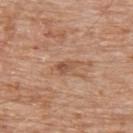The lesion was photographed on a routine skin check and not biopsied; there is no pathology result.
Cropped from a total-body skin-imaging series; the visible field is about 15 mm.
On the upper back.
A male subject approximately 80 years of age.